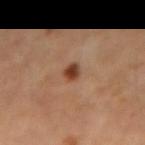Case summary:
- biopsy status — no biopsy performed (imaged during a skin exam)
- lighting — cross-polarized illumination
- acquisition — total-body-photography crop, ~15 mm field of view
- subject — female, about 60 years old
- lesion size — ~2 mm (longest diameter)
- TBP lesion metrics — an area of roughly 3.5 mm², an outline eccentricity of about 0.5 (0 = round, 1 = elongated), and two-axis asymmetry of about 0.2; border irregularity of about 1.5 on a 0–10 scale, a color-variation rating of about 3.5/10, and radial color variation of about 1
- site — the left forearm